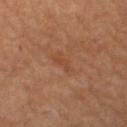{
  "automated_metrics": {
    "nevus_likeness_0_100": 0,
    "lesion_detection_confidence_0_100": 100
  },
  "site": "left thigh",
  "patient": {
    "sex": "female",
    "age_approx": 65
  },
  "image": {
    "source": "total-body photography crop",
    "field_of_view_mm": 15
  }
}